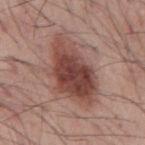Impression: This lesion was catalogued during total-body skin photography and was not selected for biopsy. Acquisition and patient details: On the mid back. Cropped from a whole-body photographic skin survey; the tile spans about 15 mm. A male subject roughly 55 years of age. Measured at roughly 8.5 mm in maximum diameter. Captured under white-light illumination.